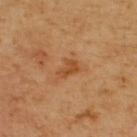notes: catalogued during a skin exam; not biopsied
image source: ~15 mm crop, total-body skin-cancer survey
location: the back
subject: male, aged approximately 45